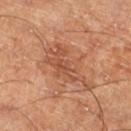follow-up = imaged on a skin check; not biopsied | body site = the left lower leg | patient = male, in their 60s | image = total-body-photography crop, ~15 mm field of view.